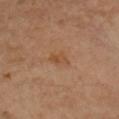{
  "patient": {
    "sex": "female",
    "age_approx": 60
  },
  "lesion_size": {
    "long_diameter_mm_approx": 2.5
  },
  "image": {
    "source": "total-body photography crop",
    "field_of_view_mm": 15
  },
  "site": "left upper arm"
}